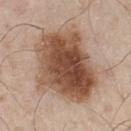Assessment:
The lesion was photographed on a routine skin check and not biopsied; there is no pathology result.
Background:
A male patient, aged 78–82. Measured at roughly 8.5 mm in maximum diameter. A close-up tile cropped from a whole-body skin photograph, about 15 mm across. From the front of the torso. Imaged with white-light lighting.